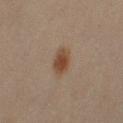Recorded during total-body skin imaging; not selected for excision or biopsy.
The lesion's longest dimension is about 3.5 mm.
A lesion tile, about 15 mm wide, cut from a 3D total-body photograph.
Captured under cross-polarized illumination.
On the right upper arm.
A male subject, about 50 years old.